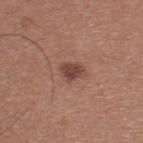notes — catalogued during a skin exam; not biopsied | location — the upper back | subject — male, aged 33 to 37 | acquisition — 15 mm crop, total-body photography | TBP lesion metrics — a footprint of about 4 mm², an eccentricity of roughly 0.6, and two-axis asymmetry of about 0.25; about 11 CIELAB-L* units darker than the surrounding skin and a normalized lesion–skin contrast near 8.5 | size — ~2.5 mm (longest diameter).